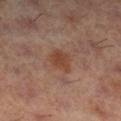| key | value |
|---|---|
| follow-up | total-body-photography surveillance lesion; no biopsy |
| site | the right lower leg |
| automated lesion analysis | a border-irregularity index near 2/10, a within-lesion color-variation index near 2/10, and radial color variation of about 0.5; an automated nevus-likeness rating near 20 out of 100 and lesion-presence confidence of about 100/100 |
| illumination | cross-polarized |
| lesion diameter | ≈3 mm |
| image | total-body-photography crop, ~15 mm field of view |
| subject | female, aged 58–62 |